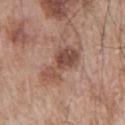biopsy_status: not biopsied; imaged during a skin examination
site: left upper arm
image:
  source: total-body photography crop
  field_of_view_mm: 15
patient:
  sex: male
  age_approx: 65